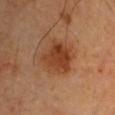| feature | finding |
|---|---|
| follow-up | total-body-photography surveillance lesion; no biopsy |
| subject | male, aged approximately 70 |
| anatomic site | the left upper arm |
| lesion diameter | ~4.5 mm (longest diameter) |
| tile lighting | cross-polarized |
| image source | ~15 mm crop, total-body skin-cancer survey |